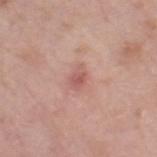notes = catalogued during a skin exam; not biopsied
image source = ~15 mm crop, total-body skin-cancer survey
body site = the right thigh
diameter = ~2.5 mm (longest diameter)
lighting = white-light illumination
patient = female, approximately 65 years of age